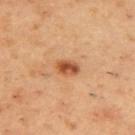<lesion>
  <biopsy_status>not biopsied; imaged during a skin examination</biopsy_status>
  <site>upper back</site>
  <automated_metrics>
    <cielab_L>52</cielab_L>
    <cielab_a>26</cielab_a>
    <cielab_b>38</cielab_b>
    <vs_skin_darker_L>13.0</vs_skin_darker_L>
    <border_irregularity_0_10>1.5</border_irregularity_0_10>
    <color_variation_0_10>4.5</color_variation_0_10>
    <peripheral_color_asymmetry>1.5</peripheral_color_asymmetry>
  </automated_metrics>
  <patient>
    <sex>male</sex>
    <age_approx>55</age_approx>
  </patient>
  <lesion_size>
    <long_diameter_mm_approx>3.0</long_diameter_mm_approx>
  </lesion_size>
  <image>
    <source>total-body photography crop</source>
    <field_of_view_mm>15</field_of_view_mm>
  </image>
</lesion>